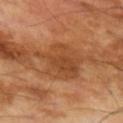lesion_size:
  long_diameter_mm_approx: 5.0
image:
  source: total-body photography crop
  field_of_view_mm: 15
patient:
  sex: male
  age_approx: 70
lighting: cross-polarized
site: left upper arm